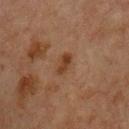This lesion was catalogued during total-body skin photography and was not selected for biopsy. A 15 mm crop from a total-body photograph taken for skin-cancer surveillance. A male subject, about 65 years old. The lesion is located on the upper back.